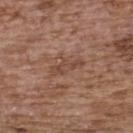| key | value |
|---|---|
| follow-up | no biopsy performed (imaged during a skin exam) |
| anatomic site | the upper back |
| patient | female, roughly 65 years of age |
| imaging modality | ~15 mm crop, total-body skin-cancer survey |